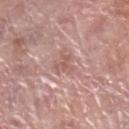Case summary:
- notes: no biopsy performed (imaged during a skin exam)
- location: the right lower leg
- patient: female, aged approximately 60
- image: ~15 mm crop, total-body skin-cancer survey